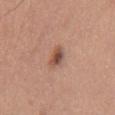Q: What is the imaging modality?
A: 15 mm crop, total-body photography
Q: Lesion location?
A: the chest
Q: How large is the lesion?
A: ~3 mm (longest diameter)
Q: What are the patient's age and sex?
A: male, in their 70s
Q: Automated lesion metrics?
A: a lesion area of about 4 mm², an eccentricity of roughly 0.85, and a shape-asymmetry score of about 0.4 (0 = symmetric); an automated nevus-likeness rating near 95 out of 100 and lesion-presence confidence of about 100/100
Q: Illumination type?
A: white-light